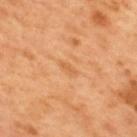biopsy status=no biopsy performed (imaged during a skin exam) | patient=male, aged 48–52 | image=total-body-photography crop, ~15 mm field of view | anatomic site=the upper back | illumination=cross-polarized illumination.